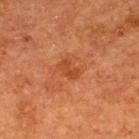Imaged during a routine full-body skin examination; the lesion was not biopsied and no histopathology is available.